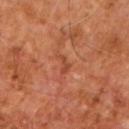Impression:
This lesion was catalogued during total-body skin photography and was not selected for biopsy.
Acquisition and patient details:
The lesion is on the right forearm. Imaged with cross-polarized lighting. An algorithmic analysis of the crop reported a lesion area of about 2.5 mm², an eccentricity of roughly 0.9, and two-axis asymmetry of about 0.55. A male subject roughly 60 years of age. A 15 mm close-up tile from a total-body photography series done for melanoma screening.